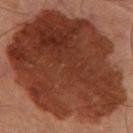Recorded during total-body skin imaging; not selected for excision or biopsy. The lesion's longest dimension is about 14.5 mm. From the right thigh. A male subject, roughly 65 years of age. Imaged with cross-polarized lighting. The total-body-photography lesion software estimated a border-irregularity index near 1.5/10, a within-lesion color-variation index near 5.5/10, and peripheral color asymmetry of about 2. And it measured an automated nevus-likeness rating near 0 out of 100 and a lesion-detection confidence of about 100/100. A lesion tile, about 15 mm wide, cut from a 3D total-body photograph.